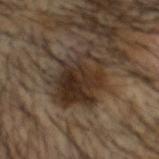Captured during whole-body skin photography for melanoma surveillance; the lesion was not biopsied.
Measured at roughly 6.5 mm in maximum diameter.
The lesion is on the head or neck.
A male patient, aged 53 to 57.
A 15 mm close-up extracted from a 3D total-body photography capture.